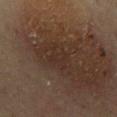| key | value |
|---|---|
| acquisition | 15 mm crop, total-body photography |
| location | the front of the torso |
| tile lighting | cross-polarized |
| subject | male, roughly 65 years of age |
| diameter | about 3 mm |
| TBP lesion metrics | an area of roughly 5 mm², a shape eccentricity near 0.65, and a shape-asymmetry score of about 0.25 (0 = symmetric); an average lesion color of about L≈25 a*≈13 b*≈20 (CIELAB), a lesion–skin lightness drop of about 4, and a normalized lesion–skin contrast near 4.5 |